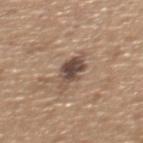{
  "biopsy_status": "not biopsied; imaged during a skin examination",
  "patient": {
    "sex": "male",
    "age_approx": 65
  },
  "image": {
    "source": "total-body photography crop",
    "field_of_view_mm": 15
  },
  "lesion_size": {
    "long_diameter_mm_approx": 3.5
  },
  "automated_metrics": {
    "cielab_L": 47,
    "cielab_a": 15,
    "cielab_b": 24,
    "vs_skin_darker_L": 13.0,
    "vs_skin_contrast_norm": 10.5
  },
  "site": "back"
}